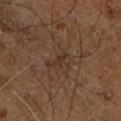The lesion was tiled from a total-body skin photograph and was not biopsied. The subject is a male about 60 years old. The lesion is located on the right leg. This is a cross-polarized tile. Measured at roughly 3 mm in maximum diameter. This image is a 15 mm lesion crop taken from a total-body photograph. Automated tile analysis of the lesion measured a lesion area of about 3 mm² and a shape-asymmetry score of about 0.5 (0 = symmetric). It also reported border irregularity of about 6.5 on a 0–10 scale and a color-variation rating of about 1/10.